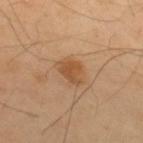Clinical summary:
Automated image analysis of the tile measured a lesion-to-skin contrast of about 7 (normalized; higher = more distinct). And it measured a border-irregularity rating of about 2.5/10, a color-variation rating of about 3.5/10, and radial color variation of about 1. And it measured a classifier nevus-likeness of about 90/100 and a lesion-detection confidence of about 100/100. This image is a 15 mm lesion crop taken from a total-body photograph. This is a cross-polarized tile. The lesion is on the upper back. Approximately 3.5 mm at its widest. The patient is a male in their 40s.